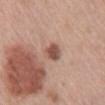Imaged during a routine full-body skin examination; the lesion was not biopsied and no histopathology is available.
The lesion is located on the mid back.
A female subject roughly 65 years of age.
Cropped from a whole-body photographic skin survey; the tile spans about 15 mm.